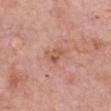tile lighting=white-light illumination | imaging modality=15 mm crop, total-body photography | location=the chest | patient=female, roughly 65 years of age | lesion diameter=~3 mm (longest diameter).